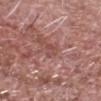The lesion was tiled from a total-body skin photograph and was not biopsied.
Cropped from a whole-body photographic skin survey; the tile spans about 15 mm.
An algorithmic analysis of the crop reported a classifier nevus-likeness of about 0/100 and a detector confidence of about 80 out of 100 that the crop contains a lesion.
The patient is a male roughly 70 years of age.
Located on the head or neck.
The tile uses white-light illumination.
Longest diameter approximately 2.5 mm.